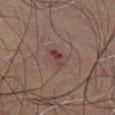The lesion was photographed on a routine skin check and not biopsied; there is no pathology result.